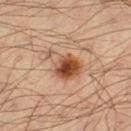| feature | finding |
|---|---|
| follow-up | imaged on a skin check; not biopsied |
| patient | male, aged 33–37 |
| lesion size | about 5 mm |
| imaging modality | 15 mm crop, total-body photography |
| site | the leg |